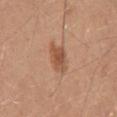- workup: total-body-photography surveillance lesion; no biopsy
- subject: male, aged around 30
- location: the mid back
- image source: 15 mm crop, total-body photography
- lesion diameter: ≈3.5 mm